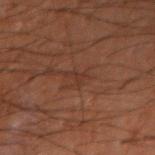Notes:
– notes · catalogued during a skin exam; not biopsied
– image · ~15 mm tile from a whole-body skin photo
– patient · male, about 70 years old
– tile lighting · cross-polarized
– TBP lesion metrics · an area of roughly 3 mm² and a shape eccentricity near 0.85; an average lesion color of about L≈25 a*≈16 b*≈21 (CIELAB), about 4 CIELAB-L* units darker than the surrounding skin, and a lesion-to-skin contrast of about 4.5 (normalized; higher = more distinct); border irregularity of about 6 on a 0–10 scale, a within-lesion color-variation index near 0/10, and radial color variation of about 0
– size · ~3 mm (longest diameter)
– body site · the left forearm